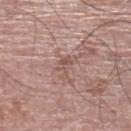follow-up: no biopsy performed (imaged during a skin exam)
image source: total-body-photography crop, ~15 mm field of view
patient: male, in their mid- to late 70s
location: the left lower leg
lesion diameter: ~3 mm (longest diameter)
automated metrics: a footprint of about 2.5 mm², an outline eccentricity of about 0.9 (0 = round, 1 = elongated), and two-axis asymmetry of about 0.75; an average lesion color of about L≈53 a*≈19 b*≈23 (CIELAB), a lesion–skin lightness drop of about 8, and a normalized border contrast of about 5.5; a border-irregularity index near 8/10 and radial color variation of about 0